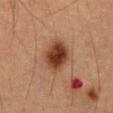Q: Was this lesion biopsied?
A: imaged on a skin check; not biopsied
Q: Automated lesion metrics?
A: an area of roughly 12 mm² and a symmetry-axis asymmetry near 0.15; a border-irregularity index near 1.5/10, internal color variation of about 5.5 on a 0–10 scale, and peripheral color asymmetry of about 1.5
Q: What is the anatomic site?
A: the mid back
Q: Patient demographics?
A: male, aged approximately 55
Q: Illumination type?
A: cross-polarized
Q: What kind of image is this?
A: 15 mm crop, total-body photography